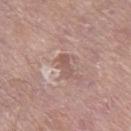follow-up: catalogued during a skin exam; not biopsied
subject: male, aged 78 to 82
diameter: ≈3 mm
lighting: white-light
site: the right thigh
image: 15 mm crop, total-body photography
automated metrics: an average lesion color of about L≈55 a*≈19 b*≈23 (CIELAB) and a normalized border contrast of about 6; a nevus-likeness score of about 0/100 and lesion-presence confidence of about 100/100